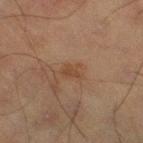Assessment:
Captured during whole-body skin photography for melanoma surveillance; the lesion was not biopsied.
Image and clinical context:
This is a cross-polarized tile. The total-body-photography lesion software estimated a footprint of about 3.5 mm², an outline eccentricity of about 0.75 (0 = round, 1 = elongated), and a symmetry-axis asymmetry near 0.3. A 15 mm close-up extracted from a 3D total-body photography capture. Measured at roughly 2.5 mm in maximum diameter. A male subject in their mid- to late 60s. On the leg.